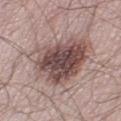Impression:
The lesion was photographed on a routine skin check and not biopsied; there is no pathology result.
Acquisition and patient details:
The lesion is on the left lower leg. A male patient, in their mid- to late 50s. Cropped from a total-body skin-imaging series; the visible field is about 15 mm.